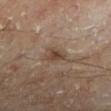Imaged during a routine full-body skin examination; the lesion was not biopsied and no histopathology is available.
The patient is aged 53 to 57.
A roughly 15 mm field-of-view crop from a total-body skin photograph.
The lesion is located on the left lower leg.
This is a cross-polarized tile.
The recorded lesion diameter is about 2.5 mm.